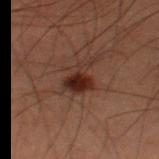| feature | finding |
|---|---|
| notes | imaged on a skin check; not biopsied |
| subject | male, aged approximately 60 |
| automated lesion analysis | a footprint of about 7.5 mm² and two-axis asymmetry of about 0.45 |
| anatomic site | the right thigh |
| image | ~15 mm tile from a whole-body skin photo |